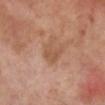Q: Was a biopsy performed?
A: imaged on a skin check; not biopsied
Q: Who is the patient?
A: female, approximately 50 years of age
Q: How was this image acquired?
A: total-body-photography crop, ~15 mm field of view
Q: What is the anatomic site?
A: the left lower leg
Q: How was the tile lit?
A: cross-polarized illumination